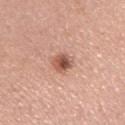Assessment: Captured during whole-body skin photography for melanoma surveillance; the lesion was not biopsied. Image and clinical context: A 15 mm close-up tile from a total-body photography series done for melanoma screening. The lesion is on the left upper arm. The subject is a female in their mid- to late 50s.